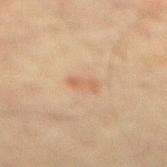{
  "biopsy_status": "not biopsied; imaged during a skin examination",
  "image": {
    "source": "total-body photography crop",
    "field_of_view_mm": 15
  },
  "automated_metrics": {
    "cielab_L": 52,
    "cielab_a": 18,
    "cielab_b": 30,
    "vs_skin_darker_L": 6.0,
    "vs_skin_contrast_norm": 5.0,
    "nevus_likeness_0_100": 5,
    "lesion_detection_confidence_0_100": 100
  },
  "lighting": "cross-polarized",
  "patient": {
    "sex": "male",
    "age_approx": 75
  },
  "lesion_size": {
    "long_diameter_mm_approx": 3.0
  },
  "site": "mid back"
}